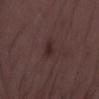The lesion was photographed on a routine skin check and not biopsied; there is no pathology result.
An algorithmic analysis of the crop reported a footprint of about 3 mm². And it measured a border-irregularity index near 2/10, a within-lesion color-variation index near 1/10, and a peripheral color-asymmetry measure near 0. The analysis additionally found a classifier nevus-likeness of about 35/100 and a lesion-detection confidence of about 100/100.
A region of skin cropped from a whole-body photographic capture, roughly 15 mm wide.
From the right thigh.
The subject is a male aged 48–52.
Imaged with white-light lighting.